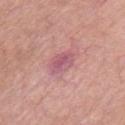Part of a total-body skin-imaging series; this lesion was reviewed on a skin check and was not flagged for biopsy. Automated tile analysis of the lesion measured an area of roughly 6 mm², an outline eccentricity of about 0.8 (0 = round, 1 = elongated), and a shape-asymmetry score of about 0.2 (0 = symmetric). It also reported a mean CIELAB color near L≈56 a*≈28 b*≈17 and about 9 CIELAB-L* units darker than the surrounding skin. It also reported a nevus-likeness score of about 0/100. About 3.5 mm across. This image is a 15 mm lesion crop taken from a total-body photograph. This is a white-light tile. The patient is a male approximately 65 years of age. The lesion is located on the chest.